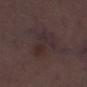<record>
<biopsy_status>not biopsied; imaged during a skin examination</biopsy_status>
<patient>
  <sex>male</sex>
  <age_approx>70</age_approx>
</patient>
<image>
  <source>total-body photography crop</source>
  <field_of_view_mm>15</field_of_view_mm>
</image>
<site>left lower leg</site>
<lighting>white-light</lighting>
</record>